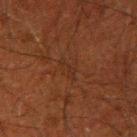Q: Was this lesion biopsied?
A: no biopsy performed (imaged during a skin exam)
Q: What is the anatomic site?
A: the right upper arm
Q: Who is the patient?
A: male, aged 48–52
Q: How was this image acquired?
A: total-body-photography crop, ~15 mm field of view
Q: How was the tile lit?
A: cross-polarized illumination
Q: Lesion size?
A: about 2.5 mm